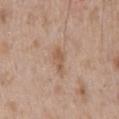Q: Patient demographics?
A: male, in their 50s
Q: What lighting was used for the tile?
A: white-light
Q: What kind of image is this?
A: ~15 mm crop, total-body skin-cancer survey
Q: What is the lesion's diameter?
A: ~3 mm (longest diameter)
Q: Lesion location?
A: the back